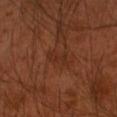biopsy status: no biopsy performed (imaged during a skin exam); patient: male, aged 48–52; anatomic site: the left arm; size: about 3 mm; image: ~15 mm crop, total-body skin-cancer survey; illumination: cross-polarized illumination.